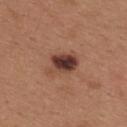notes: catalogued during a skin exam; not biopsied
imaging modality: ~15 mm crop, total-body skin-cancer survey
tile lighting: white-light illumination
subject: female, aged around 30
location: the upper back